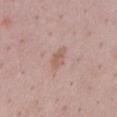Imaged during a routine full-body skin examination; the lesion was not biopsied and no histopathology is available. On the chest. Automated tile analysis of the lesion measured an average lesion color of about L≈59 a*≈19 b*≈27 (CIELAB) and a normalized lesion–skin contrast near 6.5. A region of skin cropped from a whole-body photographic capture, roughly 15 mm wide. A female subject, aged around 45.